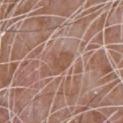Impression: Imaged during a routine full-body skin examination; the lesion was not biopsied and no histopathology is available. Acquisition and patient details: A male subject in their 60s. The recorded lesion diameter is about 2.5 mm. The tile uses white-light illumination. The lesion is located on the upper back. A region of skin cropped from a whole-body photographic capture, roughly 15 mm wide.